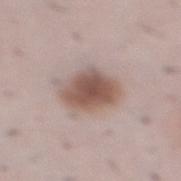Assessment: Imaged during a routine full-body skin examination; the lesion was not biopsied and no histopathology is available. Acquisition and patient details: Located on the abdomen. A male subject, aged approximately 40. The tile uses white-light illumination. A lesion tile, about 15 mm wide, cut from a 3D total-body photograph. The lesion's longest dimension is about 5 mm. Automated tile analysis of the lesion measured a color-variation rating of about 4/10. The analysis additionally found a nevus-likeness score of about 95/100 and a detector confidence of about 100 out of 100 that the crop contains a lesion.